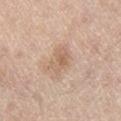Part of a total-body skin-imaging series; this lesion was reviewed on a skin check and was not flagged for biopsy.
The lesion is on the leg.
The subject is a male approximately 70 years of age.
Automated tile analysis of the lesion measured a symmetry-axis asymmetry near 0.35.
This is a white-light tile.
A close-up tile cropped from a whole-body skin photograph, about 15 mm across.
The lesion's longest dimension is about 3 mm.